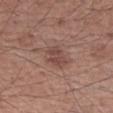The lesion was photographed on a routine skin check and not biopsied; there is no pathology result. Located on the arm. A male subject, about 60 years old. A roughly 15 mm field-of-view crop from a total-body skin photograph. The lesion-visualizer software estimated a color-variation rating of about 3/10. The analysis additionally found a nevus-likeness score of about 15/100 and a detector confidence of about 100 out of 100 that the crop contains a lesion. Imaged with white-light lighting. The lesion's longest dimension is about 3 mm.